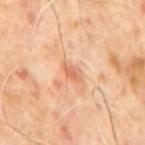Recorded during total-body skin imaging; not selected for excision or biopsy. From the mid back. A close-up tile cropped from a whole-body skin photograph, about 15 mm across. A male subject roughly 65 years of age.